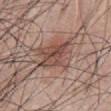{
  "biopsy_status": "not biopsied; imaged during a skin examination",
  "site": "abdomen",
  "patient": {
    "sex": "male",
    "age_approx": 45
  },
  "image": {
    "source": "total-body photography crop",
    "field_of_view_mm": 15
  },
  "automated_metrics": {
    "area_mm2_approx": 15.0,
    "eccentricity": 0.7,
    "shape_asymmetry": 0.3,
    "border_irregularity_0_10": 4.0,
    "color_variation_0_10": 6.5,
    "peripheral_color_asymmetry": 2.5
  },
  "lighting": "white-light",
  "lesion_size": {
    "long_diameter_mm_approx": 5.0
  }
}